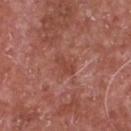Q: Was a biopsy performed?
A: catalogued during a skin exam; not biopsied
Q: How large is the lesion?
A: about 3 mm
Q: Illumination type?
A: white-light illumination
Q: Patient demographics?
A: male, in their mid-60s
Q: What kind of image is this?
A: ~15 mm crop, total-body skin-cancer survey
Q: Lesion location?
A: the chest